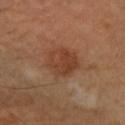<tbp_lesion>
  <patient>
    <sex>male</sex>
    <age_approx>55</age_approx>
  </patient>
  <site>right forearm</site>
  <lesion_size>
    <long_diameter_mm_approx>4.0</long_diameter_mm_approx>
  </lesion_size>
  <lighting>cross-polarized</lighting>
  <image>
    <source>total-body photography crop</source>
    <field_of_view_mm>15</field_of_view_mm>
  </image>
</tbp_lesion>